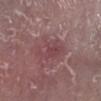{
  "patient": {
    "sex": "male",
    "age_approx": 65
  },
  "image": {
    "source": "total-body photography crop",
    "field_of_view_mm": 15
  },
  "site": "left lower leg"
}